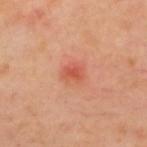• follow-up — imaged on a skin check; not biopsied
• automated metrics — a border-irregularity rating of about 1.5/10 and internal color variation of about 3.5 on a 0–10 scale; an automated nevus-likeness rating near 0 out of 100 and a detector confidence of about 100 out of 100 that the crop contains a lesion
• lesion diameter — about 2.5 mm
• image — ~15 mm crop, total-body skin-cancer survey
• patient — male, about 65 years old
• location — the upper back
• tile lighting — cross-polarized illumination